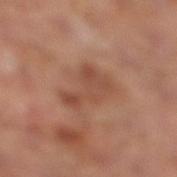Case summary:
* workup — catalogued during a skin exam; not biopsied
* imaging modality — 15 mm crop, total-body photography
* lighting — cross-polarized
* lesion size — ~5 mm (longest diameter)
* site — the right lower leg
* patient — male, aged approximately 65
* image-analysis metrics — an eccentricity of roughly 0.85; a lesion–skin lightness drop of about 7 and a lesion-to-skin contrast of about 6 (normalized; higher = more distinct); a border-irregularity index near 7/10, internal color variation of about 3 on a 0–10 scale, and a peripheral color-asymmetry measure near 1; lesion-presence confidence of about 100/100